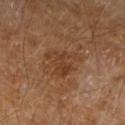Impression:
No biopsy was performed on this lesion — it was imaged during a full skin examination and was not determined to be concerning.
Image and clinical context:
This is a cross-polarized tile. About 4 mm across. The lesion is on the right forearm. The subject is a male about 85 years old or older. A 15 mm close-up extracted from a 3D total-body photography capture.